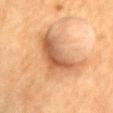Part of a total-body skin-imaging series; this lesion was reviewed on a skin check and was not flagged for biopsy. This is a cross-polarized tile. Cropped from a total-body skin-imaging series; the visible field is about 15 mm. The lesion is located on the front of the torso. The patient is a male aged approximately 60.